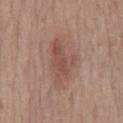No biopsy was performed on this lesion — it was imaged during a full skin examination and was not determined to be concerning. From the mid back. A 15 mm crop from a total-body photograph taken for skin-cancer surveillance. A male patient aged around 60. Measured at roughly 5.5 mm in maximum diameter.